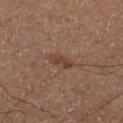Clinical impression: Recorded during total-body skin imaging; not selected for excision or biopsy. Background: This is a cross-polarized tile. A 15 mm crop from a total-body photograph taken for skin-cancer surveillance. Automated tile analysis of the lesion measured a lesion color around L≈35 a*≈17 b*≈24 in CIELAB, a lesion–skin lightness drop of about 7, and a normalized border contrast of about 6.5. The analysis additionally found a border-irregularity rating of about 3.5/10 and radial color variation of about 0. The analysis additionally found a classifier nevus-likeness of about 5/100 and lesion-presence confidence of about 100/100. The lesion is on the left lower leg. About 3 mm across. The patient is a male roughly 50 years of age.